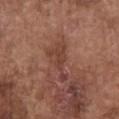{
  "patient": {
    "sex": "male",
    "age_approx": 75
  },
  "lighting": "white-light",
  "image": {
    "source": "total-body photography crop",
    "field_of_view_mm": 15
  },
  "site": "chest"
}